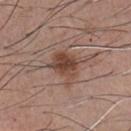{"biopsy_status": "not biopsied; imaged during a skin examination", "image": {"source": "total-body photography crop", "field_of_view_mm": 15}, "lighting": "white-light", "site": "front of the torso", "patient": {"sex": "male", "age_approx": 55}, "automated_metrics": {"eccentricity": 0.6, "cielab_L": 45, "cielab_a": 19, "cielab_b": 26, "vs_skin_darker_L": 11.0, "color_variation_0_10": 5.0, "peripheral_color_asymmetry": 2.0}, "lesion_size": {"long_diameter_mm_approx": 4.0}}